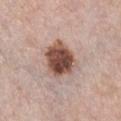Impression: The lesion was tiled from a total-body skin photograph and was not biopsied. Clinical summary: A lesion tile, about 15 mm wide, cut from a 3D total-body photograph. The lesion is on the front of the torso. A female subject in their mid-60s.